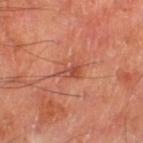{"image": {"source": "total-body photography crop", "field_of_view_mm": 15}, "site": "left thigh", "lighting": "cross-polarized", "patient": {"sex": "male", "age_approx": 70}, "lesion_size": {"long_diameter_mm_approx": 3.0}, "automated_metrics": {"eccentricity": 0.8, "cielab_L": 49, "cielab_a": 29, "cielab_b": 32, "vs_skin_darker_L": 8.0, "vs_skin_contrast_norm": 6.0}}